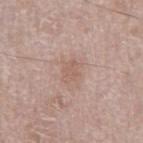  site: right thigh
  lesion_size:
    long_diameter_mm_approx: 3.0
  image:
    source: total-body photography crop
    field_of_view_mm: 15
  patient:
    sex: female
    age_approx: 75
  automated_metrics:
    eccentricity: 0.65
    shape_asymmetry: 0.3
    border_irregularity_0_10: 3.0
    color_variation_0_10: 1.5
    peripheral_color_asymmetry: 0.5
  lighting: white-light